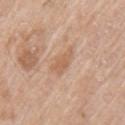This lesion was catalogued during total-body skin photography and was not selected for biopsy. The lesion is located on the left upper arm. This is a white-light tile. Automated tile analysis of the lesion measured an average lesion color of about L≈61 a*≈20 b*≈33 (CIELAB). The analysis additionally found a nevus-likeness score of about 0/100 and lesion-presence confidence of about 100/100. A region of skin cropped from a whole-body photographic capture, roughly 15 mm wide. A male patient, roughly 65 years of age.